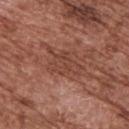  biopsy_status: not biopsied; imaged during a skin examination
  image:
    source: total-body photography crop
    field_of_view_mm: 15
  site: upper back
  patient:
    sex: male
    age_approx: 75
  lesion_size:
    long_diameter_mm_approx: 4.5
  lighting: white-light